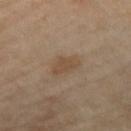Q: Is there a histopathology result?
A: imaged on a skin check; not biopsied
Q: What lighting was used for the tile?
A: cross-polarized
Q: Lesion location?
A: the left thigh
Q: Patient demographics?
A: female, aged 73–77
Q: What kind of image is this?
A: ~15 mm crop, total-body skin-cancer survey
Q: What did automated image analysis measure?
A: an area of roughly 6.5 mm², a shape eccentricity near 0.8, and a shape-asymmetry score of about 0.3 (0 = symmetric); border irregularity of about 3 on a 0–10 scale and a peripheral color-asymmetry measure near 0.5; lesion-presence confidence of about 100/100
Q: How large is the lesion?
A: about 4 mm